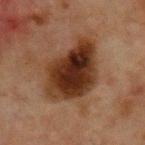Assessment:
The lesion was tiled from a total-body skin photograph and was not biopsied.
Clinical summary:
Located on the front of the torso. The lesion's longest dimension is about 7.5 mm. A male patient aged around 65. Cropped from a whole-body photographic skin survey; the tile spans about 15 mm. Automated image analysis of the tile measured a lesion–skin lightness drop of about 13 and a normalized lesion–skin contrast near 13.5. The software also gave a border-irregularity rating of about 2/10, a color-variation rating of about 7.5/10, and peripheral color asymmetry of about 2.5. And it measured an automated nevus-likeness rating near 95 out of 100.